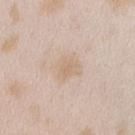Approximately 3.5 mm at its widest. A close-up tile cropped from a whole-body skin photograph, about 15 mm across. From the right forearm. This is a white-light tile. A female subject aged 23 to 27.